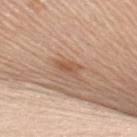| feature | finding |
|---|---|
| follow-up | total-body-photography surveillance lesion; no biopsy |
| image | ~15 mm tile from a whole-body skin photo |
| tile lighting | white-light |
| subject | female, approximately 65 years of age |
| location | the right upper arm |
| diameter | ≈3 mm |